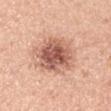Impression:
Recorded during total-body skin imaging; not selected for excision or biopsy.
Image and clinical context:
A male patient about 45 years old. Cropped from a whole-body photographic skin survey; the tile spans about 15 mm. This is a white-light tile. Longest diameter approximately 5.5 mm. The lesion is located on the arm.